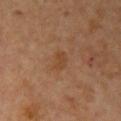This lesion was catalogued during total-body skin photography and was not selected for biopsy.
A female subject aged around 70.
A region of skin cropped from a whole-body photographic capture, roughly 15 mm wide.
Located on the arm.